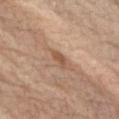Assessment:
This lesion was catalogued during total-body skin photography and was not selected for biopsy.
Clinical summary:
A 15 mm close-up extracted from a 3D total-body photography capture. About 3 mm across. An algorithmic analysis of the crop reported a lesion color around L≈54 a*≈20 b*≈32 in CIELAB and a lesion–skin lightness drop of about 9. The analysis additionally found a nevus-likeness score of about 0/100 and a lesion-detection confidence of about 90/100. Captured under white-light illumination. A male subject, in their 70s. The lesion is on the right forearm.